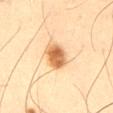The lesion was photographed on a routine skin check and not biopsied; there is no pathology result. Automated image analysis of the tile measured a mean CIELAB color near L≈54 a*≈19 b*≈36, a lesion–skin lightness drop of about 14, and a lesion-to-skin contrast of about 10 (normalized; higher = more distinct). It also reported an automated nevus-likeness rating near 100 out of 100 and lesion-presence confidence of about 100/100. A region of skin cropped from a whole-body photographic capture, roughly 15 mm wide. From the abdomen. About 3 mm across. Imaged with cross-polarized lighting. The subject is a male aged 63–67.